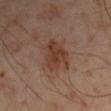Assessment:
Captured during whole-body skin photography for melanoma surveillance; the lesion was not biopsied.
Context:
The patient is a male aged 48 to 52. A region of skin cropped from a whole-body photographic capture, roughly 15 mm wide. The lesion is located on the left arm.